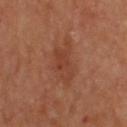Case summary:
– follow-up · catalogued during a skin exam; not biopsied
– body site · the upper back
– imaging modality · total-body-photography crop, ~15 mm field of view
– image-analysis metrics · an area of roughly 12 mm², an eccentricity of roughly 0.8, and two-axis asymmetry of about 0.35; a border-irregularity rating of about 4.5/10, internal color variation of about 3 on a 0–10 scale, and peripheral color asymmetry of about 1
– subject · male, in their 70s
– lighting · cross-polarized